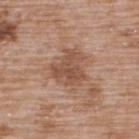Q: What is the anatomic site?
A: the back
Q: How was this image acquired?
A: 15 mm crop, total-body photography
Q: Illumination type?
A: white-light illumination
Q: Lesion size?
A: ≈4 mm
Q: What did automated image analysis measure?
A: a border-irregularity index near 5/10, internal color variation of about 3 on a 0–10 scale, and a peripheral color-asymmetry measure near 1; a nevus-likeness score of about 0/100 and lesion-presence confidence of about 100/100
Q: Who is the patient?
A: male, approximately 50 years of age